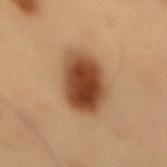Assessment: Part of a total-body skin-imaging series; this lesion was reviewed on a skin check and was not flagged for biopsy. Acquisition and patient details: Located on the mid back. A male patient aged approximately 55. The recorded lesion diameter is about 6.5 mm. Cropped from a whole-body photographic skin survey; the tile spans about 15 mm. The tile uses cross-polarized illumination.